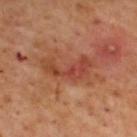The lesion was photographed on a routine skin check and not biopsied; there is no pathology result. A male patient, aged around 55. Cropped from a total-body skin-imaging series; the visible field is about 15 mm. The lesion-visualizer software estimated a lesion color around L≈43 a*≈28 b*≈32 in CIELAB, a lesion–skin lightness drop of about 9, and a normalized lesion–skin contrast near 6.5. The analysis additionally found lesion-presence confidence of about 100/100. The recorded lesion diameter is about 6 mm. Located on the back.